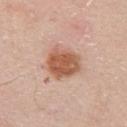Findings:
* follow-up: imaged on a skin check; not biopsied
* patient: male, in their 60s
* image source: ~15 mm tile from a whole-body skin photo
* site: the chest